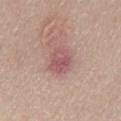The lesion was tiled from a total-body skin photograph and was not biopsied.
A male patient aged around 40.
The lesion is located on the lower back.
Cropped from a whole-body photographic skin survey; the tile spans about 15 mm.